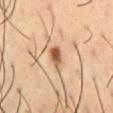The lesion was tiled from a total-body skin photograph and was not biopsied. A male subject, approximately 55 years of age. Measured at roughly 3 mm in maximum diameter. The lesion is located on the front of the torso. This is a cross-polarized tile. A 15 mm close-up extracted from a 3D total-body photography capture.